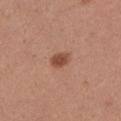| field | value |
|---|---|
| biopsy status | imaged on a skin check; not biopsied |
| location | the arm |
| patient | male, approximately 35 years of age |
| lighting | white-light illumination |
| acquisition | ~15 mm tile from a whole-body skin photo |